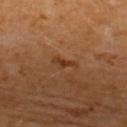Q: Is there a histopathology result?
A: catalogued during a skin exam; not biopsied
Q: What kind of image is this?
A: total-body-photography crop, ~15 mm field of view
Q: Illumination type?
A: cross-polarized
Q: Lesion location?
A: the upper back
Q: How large is the lesion?
A: ≈3 mm
Q: Automated lesion metrics?
A: a normalized border contrast of about 6.5; a border-irregularity index near 5/10, a within-lesion color-variation index near 1.5/10, and a peripheral color-asymmetry measure near 0.5; lesion-presence confidence of about 100/100
Q: What are the patient's age and sex?
A: female, aged approximately 50